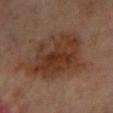Impression:
Part of a total-body skin-imaging series; this lesion was reviewed on a skin check and was not flagged for biopsy.
Background:
The lesion's longest dimension is about 8.5 mm. The lesion is located on the left lower leg. This is a cross-polarized tile. The lesion-visualizer software estimated an area of roughly 35 mm² and an outline eccentricity of about 0.7 (0 = round, 1 = elongated). It also reported a lesion–skin lightness drop of about 9 and a lesion-to-skin contrast of about 8.5 (normalized; higher = more distinct). The analysis additionally found a border-irregularity rating of about 3.5/10 and radial color variation of about 1.5. A 15 mm crop from a total-body photograph taken for skin-cancer surveillance. A male subject, aged around 70.